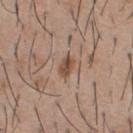Imaged during a routine full-body skin examination; the lesion was not biopsied and no histopathology is available.